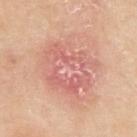Notes:
* workup — no biopsy performed (imaged during a skin exam)
* acquisition — 15 mm crop, total-body photography
* site — the left upper arm
* lighting — white-light illumination
* subject — female, aged around 70